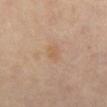{"biopsy_status": "not biopsied; imaged during a skin examination", "lesion_size": {"long_diameter_mm_approx": 2.5}, "image": {"source": "total-body photography crop", "field_of_view_mm": 15}, "patient": {"sex": "female", "age_approx": 50}, "lighting": "cross-polarized", "site": "abdomen"}